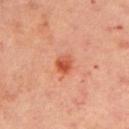A 15 mm close-up tile from a total-body photography series done for melanoma screening. A female subject, aged around 40. The lesion is located on the left upper arm.